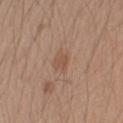follow-up — total-body-photography surveillance lesion; no biopsy
patient — male, approximately 20 years of age
image — ~15 mm tile from a whole-body skin photo
tile lighting — white-light
location — the right upper arm
automated metrics — a lesion area of about 4.5 mm², an eccentricity of roughly 0.7, and two-axis asymmetry of about 0.2; an average lesion color of about L≈52 a*≈17 b*≈29 (CIELAB), roughly 7 lightness units darker than nearby skin, and a lesion-to-skin contrast of about 5.5 (normalized; higher = more distinct); a border-irregularity rating of about 2/10, internal color variation of about 2 on a 0–10 scale, and peripheral color asymmetry of about 1
diameter — about 3 mm